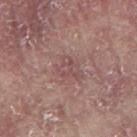Q: Was this lesion biopsied?
A: no biopsy performed (imaged during a skin exam)
Q: How was this image acquired?
A: total-body-photography crop, ~15 mm field of view
Q: What did automated image analysis measure?
A: an average lesion color of about L≈49 a*≈21 b*≈19 (CIELAB); a border-irregularity index near 5.5/10 and peripheral color asymmetry of about 1
Q: How was the tile lit?
A: white-light illumination
Q: Patient demographics?
A: male, aged 63 to 67
Q: Lesion location?
A: the right upper arm